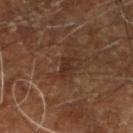Clinical impression:
Recorded during total-body skin imaging; not selected for excision or biopsy.
Clinical summary:
This is a cross-polarized tile. The total-body-photography lesion software estimated a footprint of about 3 mm² and a shape eccentricity near 0.8. The analysis additionally found a classifier nevus-likeness of about 0/100 and lesion-presence confidence of about 95/100. The recorded lesion diameter is about 2.5 mm. From the right leg. A region of skin cropped from a whole-body photographic capture, roughly 15 mm wide. A male patient aged approximately 60.